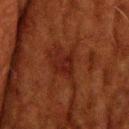Impression: The lesion was tiled from a total-body skin photograph and was not biopsied. Context: A male subject aged approximately 60. Cropped from a whole-body photographic skin survey; the tile spans about 15 mm. Located on the head or neck.